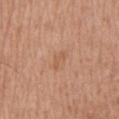Case summary:
• biopsy status: catalogued during a skin exam; not biopsied
• TBP lesion metrics: an eccentricity of roughly 0.9 and a shape-asymmetry score of about 0.35 (0 = symmetric); a classifier nevus-likeness of about 0/100 and a lesion-detection confidence of about 100/100
• patient: male, in their mid- to late 70s
• diameter: about 2.5 mm
• image: ~15 mm crop, total-body skin-cancer survey
• anatomic site: the abdomen